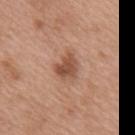Assessment:
The lesion was photographed on a routine skin check and not biopsied; there is no pathology result.
Acquisition and patient details:
Located on the mid back. A male patient about 50 years old. A region of skin cropped from a whole-body photographic capture, roughly 15 mm wide. This is a white-light tile.